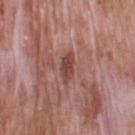Recorded during total-body skin imaging; not selected for excision or biopsy. Longest diameter approximately 3.5 mm. The lesion-visualizer software estimated an average lesion color of about L≈45 a*≈24 b*≈25 (CIELAB), roughly 10 lightness units darker than nearby skin, and a normalized lesion–skin contrast near 7.5. The analysis additionally found a color-variation rating of about 2.5/10 and peripheral color asymmetry of about 1. The software also gave an automated nevus-likeness rating near 0 out of 100 and lesion-presence confidence of about 100/100. A male subject, aged 58–62. From the back. A 15 mm close-up tile from a total-body photography series done for melanoma screening. This is a white-light tile.